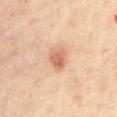A female patient, in their mid-40s.
Longest diameter approximately 2.5 mm.
The lesion is on the abdomen.
Cropped from a total-body skin-imaging series; the visible field is about 15 mm.
This is a cross-polarized tile.